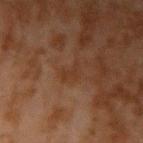Clinical impression:
No biopsy was performed on this lesion — it was imaged during a full skin examination and was not determined to be concerning.
Acquisition and patient details:
About 3 mm across. A close-up tile cropped from a whole-body skin photograph, about 15 mm across. Imaged with cross-polarized lighting. The lesion is located on the right upper arm. The subject is a male in their mid-40s.